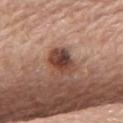Q: Is there a histopathology result?
A: total-body-photography surveillance lesion; no biopsy
Q: What is the lesion's diameter?
A: ≈3.5 mm
Q: What is the anatomic site?
A: the upper back
Q: What lighting was used for the tile?
A: white-light illumination
Q: What are the patient's age and sex?
A: female, aged around 65
Q: How was this image acquired?
A: 15 mm crop, total-body photography